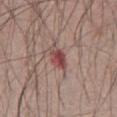Notes:
• notes · imaged on a skin check; not biopsied
• subject · male, aged 63–67
• image · 15 mm crop, total-body photography
• lesion size · about 3 mm
• body site · the abdomen
• image-analysis metrics · an average lesion color of about L≈47 a*≈25 b*≈21 (CIELAB), a lesion–skin lightness drop of about 11, and a normalized border contrast of about 8.5; a classifier nevus-likeness of about 15/100